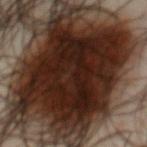Clinical impression: The lesion was tiled from a total-body skin photograph and was not biopsied. Image and clinical context: Cropped from a total-body skin-imaging series; the visible field is about 15 mm. The tile uses cross-polarized illumination. On the chest. A male patient in their mid-60s. Automated image analysis of the tile measured a lesion–skin lightness drop of about 20 and a normalized border contrast of about 19.5. The software also gave a border-irregularity index near 4/10, a within-lesion color-variation index near 6/10, and a peripheral color-asymmetry measure near 2. The lesion's longest dimension is about 13 mm.